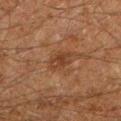Part of a total-body skin-imaging series; this lesion was reviewed on a skin check and was not flagged for biopsy. A close-up tile cropped from a whole-body skin photograph, about 15 mm across. Imaged with cross-polarized lighting. The lesion is on the left lower leg. A male subject roughly 60 years of age.